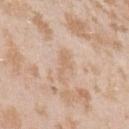The lesion was tiled from a total-body skin photograph and was not biopsied.
Approximately 3.5 mm at its widest.
From the left upper arm.
A female subject roughly 25 years of age.
A close-up tile cropped from a whole-body skin photograph, about 15 mm across.